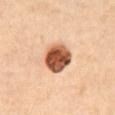Assessment: The lesion was photographed on a routine skin check and not biopsied; there is no pathology result. Background: This is a cross-polarized tile. The lesion is on the abdomen. Approximately 3.5 mm at its widest. A lesion tile, about 15 mm wide, cut from a 3D total-body photograph. The subject is a female in their mid-50s. Automated tile analysis of the lesion measured a lesion area of about 11 mm², a shape eccentricity near 0.3, and two-axis asymmetry of about 0.1. It also reported border irregularity of about 1 on a 0–10 scale, a within-lesion color-variation index near 6.5/10, and peripheral color asymmetry of about 2. And it measured a classifier nevus-likeness of about 100/100 and lesion-presence confidence of about 100/100.